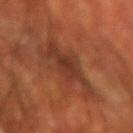follow-up: total-body-photography surveillance lesion; no biopsy
patient: male, approximately 70 years of age
site: the left forearm
lesion size: ~6.5 mm (longest diameter)
image source: total-body-photography crop, ~15 mm field of view
lighting: cross-polarized illumination